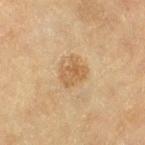{
  "biopsy_status": "not biopsied; imaged during a skin examination",
  "image": {
    "source": "total-body photography crop",
    "field_of_view_mm": 15
  },
  "automated_metrics": {
    "border_irregularity_0_10": 3.5,
    "peripheral_color_asymmetry": 1.5,
    "nevus_likeness_0_100": 20,
    "lesion_detection_confidence_0_100": 100
  },
  "patient": {
    "sex": "female",
    "age_approx": 80
  },
  "site": "left thigh",
  "lighting": "cross-polarized",
  "lesion_size": {
    "long_diameter_mm_approx": 3.0
  }
}